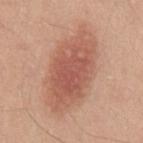{"biopsy_status": "not biopsied; imaged during a skin examination", "lesion_size": {"long_diameter_mm_approx": 10.0}, "image": {"source": "total-body photography crop", "field_of_view_mm": 15}, "site": "mid back", "patient": {"sex": "male", "age_approx": 45}, "lighting": "white-light"}